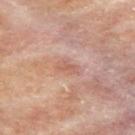The lesion was photographed on a routine skin check and not biopsied; there is no pathology result.